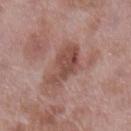Assessment:
This lesion was catalogued during total-body skin photography and was not selected for biopsy.
Acquisition and patient details:
A female subject, in their 70s. Captured under white-light illumination. Cropped from a whole-body photographic skin survey; the tile spans about 15 mm. From the left lower leg. The lesion's longest dimension is about 5 mm.